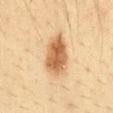Approximately 5 mm at its widest. Imaged with cross-polarized lighting. The patient is a male approximately 35 years of age. Cropped from a whole-body photographic skin survey; the tile spans about 15 mm. On the front of the torso.